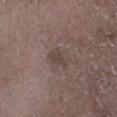Impression:
This lesion was catalogued during total-body skin photography and was not selected for biopsy.
Background:
Approximately 3 mm at its widest. A lesion tile, about 15 mm wide, cut from a 3D total-body photograph. Located on the leg. A male subject aged 68 to 72. Captured under white-light illumination.